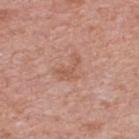biopsy_status: not biopsied; imaged during a skin examination
lighting: white-light
image:
  source: total-body photography crop
  field_of_view_mm: 15
lesion_size:
  long_diameter_mm_approx: 3.5
site: upper back
patient:
  sex: female
  age_approx: 60